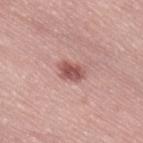– follow-up — catalogued during a skin exam; not biopsied